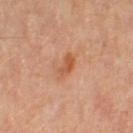Imaged during a routine full-body skin examination; the lesion was not biopsied and no histopathology is available. This is a cross-polarized tile. An algorithmic analysis of the crop reported a lesion area of about 4.5 mm² and an eccentricity of roughly 0.85. And it measured a mean CIELAB color near L≈54 a*≈26 b*≈35, roughly 9 lightness units darker than nearby skin, and a lesion-to-skin contrast of about 7 (normalized; higher = more distinct). The patient is a female aged 48–52. On the left thigh. The lesion's longest dimension is about 3 mm. A lesion tile, about 15 mm wide, cut from a 3D total-body photograph.